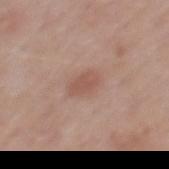Clinical impression:
Captured during whole-body skin photography for melanoma surveillance; the lesion was not biopsied.
Image and clinical context:
The lesion is located on the mid back. A lesion tile, about 15 mm wide, cut from a 3D total-body photograph. Imaged with white-light lighting. The lesion's longest dimension is about 3 mm. A male subject, about 55 years old.